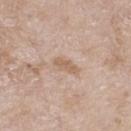Clinical impression: No biopsy was performed on this lesion — it was imaged during a full skin examination and was not determined to be concerning. Acquisition and patient details: The lesion is on the left thigh. The total-body-photography lesion software estimated a lesion area of about 4 mm², an outline eccentricity of about 0.9 (0 = round, 1 = elongated), and a shape-asymmetry score of about 0.25 (0 = symmetric). Cropped from a total-body skin-imaging series; the visible field is about 15 mm. A female subject about 75 years old.